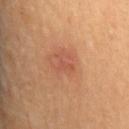• biopsy status · imaged on a skin check; not biopsied